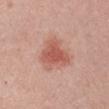Recorded during total-body skin imaging; not selected for excision or biopsy. A male subject approximately 40 years of age. From the right upper arm. A lesion tile, about 15 mm wide, cut from a 3D total-body photograph. An algorithmic analysis of the crop reported a lesion area of about 15 mm², an outline eccentricity of about 0.5 (0 = round, 1 = elongated), and a shape-asymmetry score of about 0.35 (0 = symmetric). The software also gave a border-irregularity rating of about 4/10. The software also gave a detector confidence of about 100 out of 100 that the crop contains a lesion. Imaged with white-light lighting. Longest diameter approximately 5 mm.